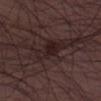* biopsy status · imaged on a skin check; not biopsied
* location · the left thigh
* image · 15 mm crop, total-body photography
* subject · male, aged approximately 50